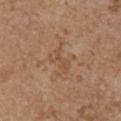follow-up = imaged on a skin check; not biopsied.